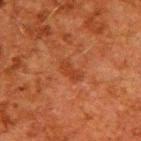Recorded during total-body skin imaging; not selected for excision or biopsy. Automated image analysis of the tile measured a shape eccentricity near 0.85 and a symmetry-axis asymmetry near 0.35. The software also gave a mean CIELAB color near L≈33 a*≈25 b*≈32, a lesion–skin lightness drop of about 5, and a normalized border contrast of about 5.5. And it measured lesion-presence confidence of about 100/100. The subject is a male about 60 years old. Captured under cross-polarized illumination. The lesion's longest dimension is about 3 mm. From the back. A close-up tile cropped from a whole-body skin photograph, about 15 mm across.